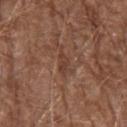follow-up = total-body-photography surveillance lesion; no biopsy | automated metrics = a footprint of about 2.5 mm², an eccentricity of roughly 0.9, and two-axis asymmetry of about 0.4 | site = the left forearm | size = about 2.5 mm | subject = male, roughly 70 years of age | imaging modality = total-body-photography crop, ~15 mm field of view | tile lighting = white-light.